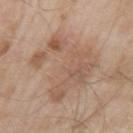follow-up — no biopsy performed (imaged during a skin exam)
acquisition — 15 mm crop, total-body photography
site — the left upper arm
patient — male, aged 53–57